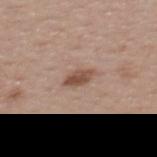workup — total-body-photography surveillance lesion; no biopsy | patient — female, roughly 55 years of age | tile lighting — white-light illumination | image — ~15 mm crop, total-body skin-cancer survey | location — the upper back.